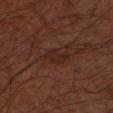This lesion was catalogued during total-body skin photography and was not selected for biopsy.
The tile uses cross-polarized illumination.
Cropped from a total-body skin-imaging series; the visible field is about 15 mm.
On the left forearm.
Automated image analysis of the tile measured an outline eccentricity of about 0.8 (0 = round, 1 = elongated) and a symmetry-axis asymmetry near 0.4.
A male patient roughly 60 years of age.